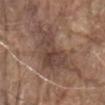Impression:
Recorded during total-body skin imaging; not selected for excision or biopsy.
Context:
Approximately 9 mm at its widest. Cropped from a whole-body photographic skin survey; the tile spans about 15 mm. The patient is a male aged approximately 80. On the abdomen. Automated image analysis of the tile measured a classifier nevus-likeness of about 5/100 and a detector confidence of about 75 out of 100 that the crop contains a lesion.